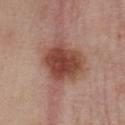biopsy status: total-body-photography surveillance lesion; no biopsy
image source: 15 mm crop, total-body photography
body site: the chest
lighting: white-light illumination
image-analysis metrics: an eccentricity of roughly 0.5 and a symmetry-axis asymmetry near 0.35; border irregularity of about 4 on a 0–10 scale, a within-lesion color-variation index near 5/10, and a peripheral color-asymmetry measure near 1
lesion diameter: about 6.5 mm
subject: female, in their mid- to late 50s